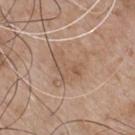image-analysis metrics=a border-irregularity rating of about 6.5/10, a within-lesion color-variation index near 3.5/10, and radial color variation of about 1; lesion-presence confidence of about 100/100 | lesion diameter=≈5 mm | image source=15 mm crop, total-body photography | location=the chest | patient=male, aged 63–67.